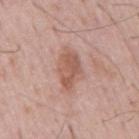Clinical impression: Imaged during a routine full-body skin examination; the lesion was not biopsied and no histopathology is available. Context: The recorded lesion diameter is about 4 mm. The patient is a male about 55 years old. Cropped from a whole-body photographic skin survey; the tile spans about 15 mm. This is a white-light tile.